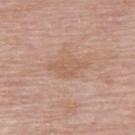A 15 mm crop from a total-body photograph taken for skin-cancer surveillance. About 4.5 mm across. From the upper back. A female subject, aged 63 to 67. The tile uses white-light illumination.